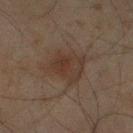Impression:
No biopsy was performed on this lesion — it was imaged during a full skin examination and was not determined to be concerning.
Background:
The subject is a male aged 43–47. Longest diameter approximately 5.5 mm. Cropped from a whole-body photographic skin survey; the tile spans about 15 mm. The tile uses cross-polarized illumination. Located on the leg.